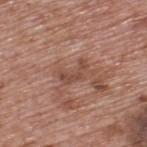Q: Is there a histopathology result?
A: imaged on a skin check; not biopsied
Q: Patient demographics?
A: male, aged 68 to 72
Q: How was this image acquired?
A: 15 mm crop, total-body photography
Q: Illumination type?
A: white-light illumination
Q: How large is the lesion?
A: ≈3 mm
Q: Where on the body is the lesion?
A: the back